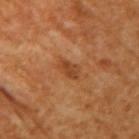Clinical impression: This lesion was catalogued during total-body skin photography and was not selected for biopsy.